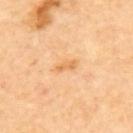<record>
<biopsy_status>not biopsied; imaged during a skin examination</biopsy_status>
<lesion_size>
  <long_diameter_mm_approx>2.5</long_diameter_mm_approx>
</lesion_size>
<site>upper back</site>
<lighting>cross-polarized</lighting>
<image>
  <source>total-body photography crop</source>
  <field_of_view_mm>15</field_of_view_mm>
</image>
</record>